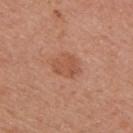Case summary:
– follow-up · total-body-photography surveillance lesion; no biopsy
– site · the right upper arm
– subject · female, in their 40s
– lighting · white-light illumination
– acquisition · 15 mm crop, total-body photography